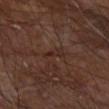Impression: No biopsy was performed on this lesion — it was imaged during a full skin examination and was not determined to be concerning. Background: Automated image analysis of the tile measured a lesion area of about 3 mm², a shape eccentricity near 0.85, and a shape-asymmetry score of about 0.4 (0 = symmetric). The analysis additionally found a mean CIELAB color near L≈25 a*≈17 b*≈21. The analysis additionally found lesion-presence confidence of about 75/100. The tile uses cross-polarized illumination. A male subject, approximately 65 years of age. Cropped from a total-body skin-imaging series; the visible field is about 15 mm. On the right forearm.